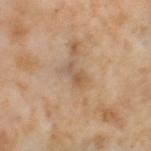This lesion was catalogued during total-body skin photography and was not selected for biopsy.